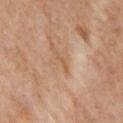notes: no biopsy performed (imaged during a skin exam)
location: the left upper arm
acquisition: total-body-photography crop, ~15 mm field of view
size: about 2.5 mm
illumination: cross-polarized
subject: female, about 50 years old